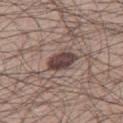Captured during whole-body skin photography for melanoma surveillance; the lesion was not biopsied. A male patient, aged 28–32. On the right thigh. This is a white-light tile. Cropped from a whole-body photographic skin survey; the tile spans about 15 mm. Approximately 4 mm at its widest.